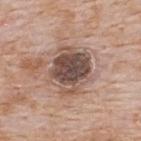Captured during whole-body skin photography for melanoma surveillance; the lesion was not biopsied.
A 15 mm close-up extracted from a 3D total-body photography capture.
The patient is a male aged approximately 65.
Imaged with white-light lighting.
Measured at roughly 5.5 mm in maximum diameter.
The lesion-visualizer software estimated an area of roughly 19 mm² and a symmetry-axis asymmetry near 0.2. The analysis additionally found a lesion color around L≈49 a*≈17 b*≈23 in CIELAB, a lesion–skin lightness drop of about 15, and a lesion-to-skin contrast of about 10.5 (normalized; higher = more distinct). The software also gave a within-lesion color-variation index near 6.5/10 and radial color variation of about 1.5. The analysis additionally found an automated nevus-likeness rating near 5 out of 100 and a lesion-detection confidence of about 100/100.
On the back.